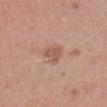Recorded during total-body skin imaging; not selected for excision or biopsy.
The patient is a female approximately 20 years of age.
A region of skin cropped from a whole-body photographic capture, roughly 15 mm wide.
The recorded lesion diameter is about 2.5 mm.
Located on the head or neck.
Imaged with white-light lighting.